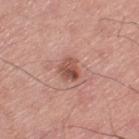{"biopsy_status": "not biopsied; imaged during a skin examination", "automated_metrics": {"eccentricity": 0.5, "shape_asymmetry": 0.3, "border_irregularity_0_10": 2.5, "peripheral_color_asymmetry": 3.0, "nevus_likeness_0_100": 55, "lesion_detection_confidence_0_100": 100}, "patient": {"sex": "male", "age_approx": 70}, "lighting": "white-light", "image": {"source": "total-body photography crop", "field_of_view_mm": 15}, "site": "left thigh"}